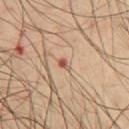workup: imaged on a skin check; not biopsied | tile lighting: cross-polarized | acquisition: ~15 mm tile from a whole-body skin photo | automated lesion analysis: internal color variation of about 0 on a 0–10 scale; a nevus-likeness score of about 25/100 and a detector confidence of about 100 out of 100 that the crop contains a lesion | location: the chest | subject: male, approximately 65 years of age.